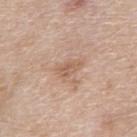Assessment: Imaged during a routine full-body skin examination; the lesion was not biopsied and no histopathology is available. Acquisition and patient details: About 3 mm across. A 15 mm close-up extracted from a 3D total-body photography capture. Located on the chest. Captured under white-light illumination. The patient is a male aged around 80.